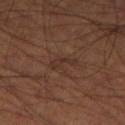Imaged during a routine full-body skin examination; the lesion was not biopsied and no histopathology is available. A male subject, roughly 70 years of age. Imaged with cross-polarized lighting. Longest diameter approximately 3 mm. Cropped from a whole-body photographic skin survey; the tile spans about 15 mm. From the leg. The lesion-visualizer software estimated a lesion-to-skin contrast of about 5.5 (normalized; higher = more distinct).